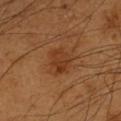biopsy_status: not biopsied; imaged during a skin examination
patient:
  sex: male
  age_approx: 60
automated_metrics:
  area_mm2_approx: 6.5
  eccentricity: 0.65
  cielab_L: 36
  cielab_a: 24
  cielab_b: 34
  vs_skin_contrast_norm: 6.5
  color_variation_0_10: 3.0
  peripheral_color_asymmetry: 1.0
  nevus_likeness_0_100: 40
  lesion_detection_confidence_0_100: 100
lighting: cross-polarized
lesion_size:
  long_diameter_mm_approx: 3.5
image:
  source: total-body photography crop
  field_of_view_mm: 15
site: left upper arm